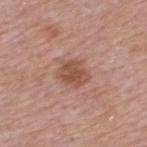Acquisition and patient details: Captured under white-light illumination. An algorithmic analysis of the crop reported border irregularity of about 2 on a 0–10 scale. And it measured a classifier nevus-likeness of about 35/100 and a lesion-detection confidence of about 100/100. The patient is a male aged around 55. Approximately 3 mm at its widest. Cropped from a total-body skin-imaging series; the visible field is about 15 mm. From the upper back.